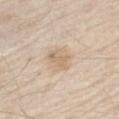workup: imaged on a skin check; not biopsied
body site: the left upper arm
imaging modality: ~15 mm crop, total-body skin-cancer survey
patient: male, aged 63 to 67